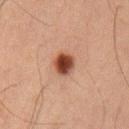Q: Was a biopsy performed?
A: total-body-photography surveillance lesion; no biopsy
Q: What lighting was used for the tile?
A: cross-polarized illumination
Q: What is the imaging modality?
A: 15 mm crop, total-body photography
Q: What is the lesion's diameter?
A: ≈3 mm
Q: Patient demographics?
A: male, approximately 50 years of age
Q: What is the anatomic site?
A: the left upper arm